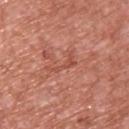The lesion was tiled from a total-body skin photograph and was not biopsied.
A male patient, roughly 55 years of age.
The lesion is located on the back.
A roughly 15 mm field-of-view crop from a total-body skin photograph.
Captured under white-light illumination.
An algorithmic analysis of the crop reported a border-irregularity index near 10/10, a within-lesion color-variation index near 0/10, and peripheral color asymmetry of about 0. The software also gave a classifier nevus-likeness of about 0/100.